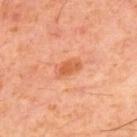| feature | finding |
|---|---|
| notes | catalogued during a skin exam; not biopsied |
| subject | male, approximately 60 years of age |
| body site | the back |
| image | 15 mm crop, total-body photography |